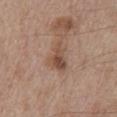{"biopsy_status": "not biopsied; imaged during a skin examination", "site": "front of the torso", "image": {"source": "total-body photography crop", "field_of_view_mm": 15}, "lighting": "white-light", "automated_metrics": {"area_mm2_approx": 5.5, "eccentricity": 0.9, "shape_asymmetry": 0.45, "border_irregularity_0_10": 5.0, "peripheral_color_asymmetry": 1.0, "nevus_likeness_0_100": 20, "lesion_detection_confidence_0_100": 100}, "patient": {"sex": "male", "age_approx": 70}, "lesion_size": {"long_diameter_mm_approx": 4.0}}